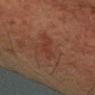{"biopsy_status": "not biopsied; imaged during a skin examination", "lesion_size": {"long_diameter_mm_approx": 3.5}, "automated_metrics": {"nevus_likeness_0_100": 10, "lesion_detection_confidence_0_100": 100}, "patient": {"sex": "male", "age_approx": 60}, "site": "left forearm", "lighting": "cross-polarized", "image": {"source": "total-body photography crop", "field_of_view_mm": 15}}